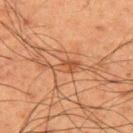Captured during whole-body skin photography for melanoma surveillance; the lesion was not biopsied. A male subject, approximately 60 years of age. A region of skin cropped from a whole-body photographic capture, roughly 15 mm wide. This is a cross-polarized tile. The lesion is on the upper back.